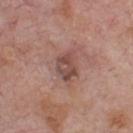biopsy status: no biopsy performed (imaged during a skin exam)
subject: male, aged around 75
body site: the chest
lighting: white-light illumination
lesion size: about 3.5 mm
imaging modality: 15 mm crop, total-body photography
automated metrics: a footprint of about 6.5 mm²; a lesion color around L≈47 a*≈21 b*≈23 in CIELAB, about 10 CIELAB-L* units darker than the surrounding skin, and a lesion-to-skin contrast of about 7.5 (normalized; higher = more distinct); a border-irregularity index near 2.5/10, internal color variation of about 4.5 on a 0–10 scale, and peripheral color asymmetry of about 1.5; a detector confidence of about 100 out of 100 that the crop contains a lesion